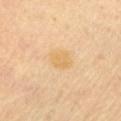Impression:
Part of a total-body skin-imaging series; this lesion was reviewed on a skin check and was not flagged for biopsy.
Image and clinical context:
The lesion-visualizer software estimated a mean CIELAB color near L≈70 a*≈16 b*≈43, roughly 6 lightness units darker than nearby skin, and a normalized border contrast of about 6. And it measured an automated nevus-likeness rating near 5 out of 100. The lesion is on the chest. This is a cross-polarized tile. The recorded lesion diameter is about 3 mm. A 15 mm close-up tile from a total-body photography series done for melanoma screening. A male patient aged approximately 70.